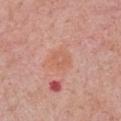{"site": "right upper arm", "patient": {"sex": "male", "age_approx": 75}, "lighting": "white-light", "lesion_size": {"long_diameter_mm_approx": 2.5}, "image": {"source": "total-body photography crop", "field_of_view_mm": 15}}